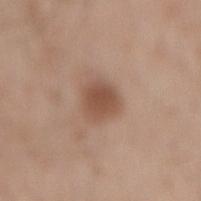| key | value |
|---|---|
| biopsy status | total-body-photography surveillance lesion; no biopsy |
| tile lighting | white-light |
| location | the back |
| image source | total-body-photography crop, ~15 mm field of view |
| automated lesion analysis | a mean CIELAB color near L≈51 a*≈19 b*≈29, a lesion–skin lightness drop of about 11, and a normalized border contrast of about 8; a border-irregularity rating of about 1.5/10, internal color variation of about 2.5 on a 0–10 scale, and a peripheral color-asymmetry measure near 0.5 |
| subject | male, roughly 60 years of age |
| diameter | ~3.5 mm (longest diameter) |